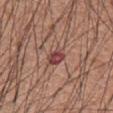Clinical summary: Imaged with white-light lighting. A lesion tile, about 15 mm wide, cut from a 3D total-body photograph. A male patient aged 58 to 62. From the chest.